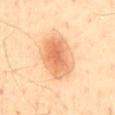{
  "biopsy_status": "not biopsied; imaged during a skin examination",
  "lesion_size": {
    "long_diameter_mm_approx": 6.0
  },
  "automated_metrics": {
    "cielab_L": 70,
    "cielab_a": 24,
    "cielab_b": 41,
    "vs_skin_darker_L": 13.0,
    "vs_skin_contrast_norm": 7.5,
    "border_irregularity_0_10": 3.0,
    "color_variation_0_10": 5.0,
    "nevus_likeness_0_100": 100,
    "lesion_detection_confidence_0_100": 100
  },
  "patient": {
    "sex": "male",
    "age_approx": 60
  },
  "lighting": "cross-polarized",
  "site": "back",
  "image": {
    "source": "total-body photography crop",
    "field_of_view_mm": 15
  }
}